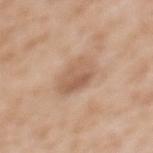| feature | finding |
|---|---|
| workup | no biopsy performed (imaged during a skin exam) |
| illumination | white-light |
| lesion size | ~4.5 mm (longest diameter) |
| subject | female, aged around 40 |
| automated lesion analysis | an area of roughly 12 mm²; a mean CIELAB color near L≈59 a*≈18 b*≈31 and a normalized border contrast of about 6; radial color variation of about 1.5 |
| acquisition | total-body-photography crop, ~15 mm field of view |
| anatomic site | the upper back |